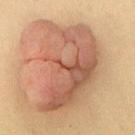Recorded during total-body skin imaging; not selected for excision or biopsy. A male patient, aged 33 to 37. Located on the upper back. A lesion tile, about 15 mm wide, cut from a 3D total-body photograph.